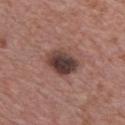Case summary:
- anatomic site: the chest
- image: ~15 mm crop, total-body skin-cancer survey
- subject: male, aged 68–72